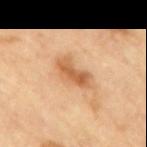- follow-up — catalogued during a skin exam; not biopsied
- image-analysis metrics — an area of roughly 8 mm² and two-axis asymmetry of about 0.35; a border-irregularity index near 4/10 and radial color variation of about 1.5; a nevus-likeness score of about 5/100 and a lesion-detection confidence of about 100/100
- image — total-body-photography crop, ~15 mm field of view
- patient — male, aged 83 or older
- body site — the mid back